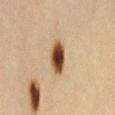| field | value |
|---|---|
| workup | catalogued during a skin exam; not biopsied |
| image source | 15 mm crop, total-body photography |
| body site | the back |
| automated lesion analysis | a footprint of about 7.5 mm², a shape eccentricity near 0.8, and two-axis asymmetry of about 0.2; a color-variation rating of about 6/10 and a peripheral color-asymmetry measure near 1.5; a detector confidence of about 100 out of 100 that the crop contains a lesion |
| lesion diameter | ~4 mm (longest diameter) |
| subject | female, aged around 45 |
| illumination | cross-polarized illumination |